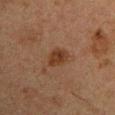{
  "biopsy_status": "not biopsied; imaged during a skin examination",
  "image": {
    "source": "total-body photography crop",
    "field_of_view_mm": 15
  },
  "lesion_size": {
    "long_diameter_mm_approx": 3.0
  },
  "patient": {
    "sex": "male",
    "age_approx": 50
  },
  "site": "left upper arm",
  "lighting": "cross-polarized"
}